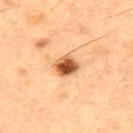Clinical impression:
No biopsy was performed on this lesion — it was imaged during a full skin examination and was not determined to be concerning.
Image and clinical context:
A male subject in their 50s. A close-up tile cropped from a whole-body skin photograph, about 15 mm across. Located on the upper back. The lesion's longest dimension is about 3 mm. This is a cross-polarized tile.